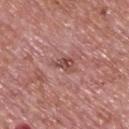Q: Was this lesion biopsied?
A: catalogued during a skin exam; not biopsied
Q: What is the lesion's diameter?
A: ~2.5 mm (longest diameter)
Q: How was this image acquired?
A: ~15 mm tile from a whole-body skin photo
Q: Where on the body is the lesion?
A: the upper back
Q: How was the tile lit?
A: white-light
Q: Automated lesion metrics?
A: about 10 CIELAB-L* units darker than the surrounding skin and a normalized lesion–skin contrast near 7
Q: What are the patient's age and sex?
A: male, aged around 75